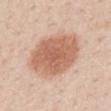{
  "biopsy_status": "not biopsied; imaged during a skin examination",
  "patient": {
    "sex": "female",
    "age_approx": 45
  },
  "lesion_size": {
    "long_diameter_mm_approx": 7.5
  },
  "lighting": "white-light",
  "site": "mid back",
  "automated_metrics": {
    "lesion_detection_confidence_0_100": 100
  },
  "image": {
    "source": "total-body photography crop",
    "field_of_view_mm": 15
  }
}